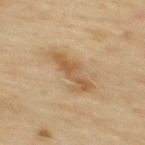notes: no biopsy performed (imaged during a skin exam) | patient: male, in their mid-40s | tile lighting: cross-polarized | location: the upper back | image-analysis metrics: a lesion area of about 8.5 mm², an eccentricity of roughly 0.9, and a shape-asymmetry score of about 0.35 (0 = symmetric); a lesion color around L≈47 a*≈14 b*≈32 in CIELAB, roughly 8 lightness units darker than nearby skin, and a normalized lesion–skin contrast near 6.5; an automated nevus-likeness rating near 10 out of 100 and a detector confidence of about 100 out of 100 that the crop contains a lesion | size: ~5 mm (longest diameter) | image source: ~15 mm tile from a whole-body skin photo.